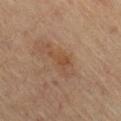<case>
<biopsy_status>not biopsied; imaged during a skin examination</biopsy_status>
<image>
  <source>total-body photography crop</source>
  <field_of_view_mm>15</field_of_view_mm>
</image>
<patient>
  <sex>female</sex>
  <age_approx>65</age_approx>
</patient>
<lesion_size>
  <long_diameter_mm_approx>3.0</long_diameter_mm_approx>
</lesion_size>
<lighting>cross-polarized</lighting>
<site>front of the torso</site>
</case>